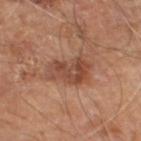Recorded during total-body skin imaging; not selected for excision or biopsy. The lesion's longest dimension is about 5 mm. Automated tile analysis of the lesion measured a footprint of about 12 mm², an eccentricity of roughly 0.75, and two-axis asymmetry of about 0.35. The software also gave a border-irregularity index near 4/10, a within-lesion color-variation index near 4.5/10, and a peripheral color-asymmetry measure near 1.5. From the left lower leg. The subject is a male aged approximately 70. Cropped from a whole-body photographic skin survey; the tile spans about 15 mm.